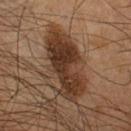{
  "biopsy_status": "not biopsied; imaged during a skin examination",
  "image": {
    "source": "total-body photography crop",
    "field_of_view_mm": 15
  },
  "lesion_size": {
    "long_diameter_mm_approx": 9.0
  },
  "site": "right lower leg",
  "patient": {
    "sex": "male",
    "age_approx": 55
  }
}